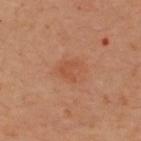Assessment:
Captured during whole-body skin photography for melanoma surveillance; the lesion was not biopsied.